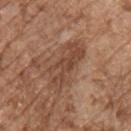Impression:
Captured during whole-body skin photography for melanoma surveillance; the lesion was not biopsied.
Image and clinical context:
A lesion tile, about 15 mm wide, cut from a 3D total-body photograph. An algorithmic analysis of the crop reported a footprint of about 14 mm², an outline eccentricity of about 0.9 (0 = round, 1 = elongated), and two-axis asymmetry of about 0.25. It also reported a nevus-likeness score of about 15/100 and a lesion-detection confidence of about 90/100. The lesion is on the chest. A male subject, aged around 75. Approximately 6.5 mm at its widest. The tile uses white-light illumination.